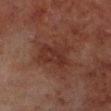Clinical impression:
This lesion was catalogued during total-body skin photography and was not selected for biopsy.
Context:
A roughly 15 mm field-of-view crop from a total-body skin photograph. The tile uses cross-polarized illumination. Located on the left lower leg. The patient is a male aged around 70. Automated tile analysis of the lesion measured an outline eccentricity of about 0.5 (0 = round, 1 = elongated) and two-axis asymmetry of about 0.35. The analysis additionally found a mean CIELAB color near L≈26 a*≈19 b*≈21, about 6 CIELAB-L* units darker than the surrounding skin, and a lesion-to-skin contrast of about 6 (normalized; higher = more distinct). It also reported a border-irregularity index near 4.5/10, a within-lesion color-variation index near 3/10, and a peripheral color-asymmetry measure near 1. It also reported an automated nevus-likeness rating near 5 out of 100 and a lesion-detection confidence of about 100/100.